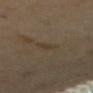{"image": {"source": "total-body photography crop", "field_of_view_mm": 15}, "site": "mid back", "patient": {"sex": "female", "age_approx": 55}, "automated_metrics": {"area_mm2_approx": 3.0, "eccentricity": 0.85, "shape_asymmetry": 0.3, "color_variation_0_10": 1.0, "peripheral_color_asymmetry": 0.5}, "lesion_size": {"long_diameter_mm_approx": 2.5}, "lighting": "cross-polarized"}